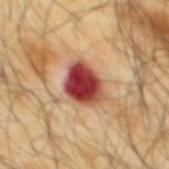A male subject, aged around 65. The tile uses cross-polarized illumination. Located on the mid back. Cropped from a whole-body photographic skin survey; the tile spans about 15 mm.